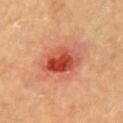This lesion was catalogued during total-body skin photography and was not selected for biopsy.
A male subject approximately 85 years of age or older.
Captured under cross-polarized illumination.
The lesion is located on the front of the torso.
A 15 mm crop from a total-body photograph taken for skin-cancer surveillance.
The lesion's longest dimension is about 5 mm.
Automated image analysis of the tile measured an area of roughly 12 mm², an eccentricity of roughly 0.75, and two-axis asymmetry of about 0.2. The analysis additionally found a lesion color around L≈50 a*≈36 b*≈36 in CIELAB and a lesion-to-skin contrast of about 9.5 (normalized; higher = more distinct).